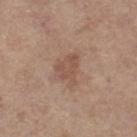follow-up: no biopsy performed (imaged during a skin exam)
TBP lesion metrics: a symmetry-axis asymmetry near 0.4; a nevus-likeness score of about 0/100 and a lesion-detection confidence of about 100/100
body site: the left lower leg
lighting: white-light illumination
subject: female, in their mid- to late 60s
imaging modality: ~15 mm crop, total-body skin-cancer survey
diameter: about 3.5 mm